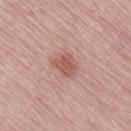Clinical impression: No biopsy was performed on this lesion — it was imaged during a full skin examination and was not determined to be concerning. Image and clinical context: About 3 mm across. Cropped from a total-body skin-imaging series; the visible field is about 15 mm. From the right thigh. The subject is a female approximately 65 years of age.